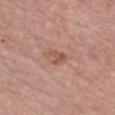Part of a total-body skin-imaging series; this lesion was reviewed on a skin check and was not flagged for biopsy.
The recorded lesion diameter is about 2.5 mm.
A close-up tile cropped from a whole-body skin photograph, about 15 mm across.
Located on the chest.
The lesion-visualizer software estimated a border-irregularity index near 4.5/10, a color-variation rating of about 1/10, and radial color variation of about 0.5. The software also gave a classifier nevus-likeness of about 0/100 and a lesion-detection confidence of about 100/100.
The subject is a female aged 63 to 67.
Captured under white-light illumination.